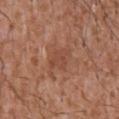Captured during whole-body skin photography for melanoma surveillance; the lesion was not biopsied. Automated tile analysis of the lesion measured a footprint of about 6 mm², a shape eccentricity near 0.7, and a shape-asymmetry score of about 0.25 (0 = symmetric). The software also gave roughly 6 lightness units darker than nearby skin and a normalized border contrast of about 4.5. The software also gave a border-irregularity index near 2.5/10, a within-lesion color-variation index near 1.5/10, and a peripheral color-asymmetry measure near 0.5. And it measured a classifier nevus-likeness of about 0/100. The subject is a male in their mid- to late 40s. From the chest. Longest diameter approximately 3 mm. The tile uses white-light illumination. A 15 mm close-up extracted from a 3D total-body photography capture.